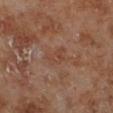Captured during whole-body skin photography for melanoma surveillance; the lesion was not biopsied. A 15 mm close-up tile from a total-body photography series done for melanoma screening. Measured at roughly 3 mm in maximum diameter. The lesion is located on the left lower leg. The total-body-photography lesion software estimated a lesion color around L≈42 a*≈20 b*≈27 in CIELAB, a lesion–skin lightness drop of about 5, and a normalized lesion–skin contrast near 5. Imaged with cross-polarized lighting. The patient is a male approximately 70 years of age.